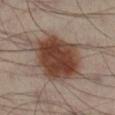biopsy_status: not biopsied; imaged during a skin examination
site: right lower leg
lighting: cross-polarized
patient:
  sex: male
  age_approx: 40
lesion_size:
  long_diameter_mm_approx: 10.0
image:
  source: total-body photography crop
  field_of_view_mm: 15